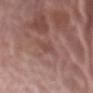follow-up = no biopsy performed (imaged during a skin exam)
patient = female, about 75 years old
illumination = white-light illumination
image source = ~15 mm tile from a whole-body skin photo
lesion size = ~2.5 mm (longest diameter)
image-analysis metrics = roughly 6 lightness units darker than nearby skin and a normalized border contrast of about 4.5; a classifier nevus-likeness of about 0/100 and a lesion-detection confidence of about 100/100
body site = the abdomen